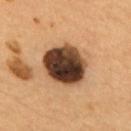Imaged during a routine full-body skin examination; the lesion was not biopsied and no histopathology is available. This is a cross-polarized tile. The lesion is on the upper back. A roughly 15 mm field-of-view crop from a total-body skin photograph. The subject is a male aged 53 to 57. Longest diameter approximately 5.5 mm.